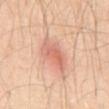Q: What is the imaging modality?
A: ~15 mm tile from a whole-body skin photo
Q: What are the patient's age and sex?
A: male, aged 53 to 57
Q: What is the lesion's diameter?
A: ≈5 mm
Q: Where on the body is the lesion?
A: the abdomen
Q: Illumination type?
A: cross-polarized